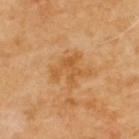{"lighting": "cross-polarized", "image": {"source": "total-body photography crop", "field_of_view_mm": 15}, "patient": {"sex": "male", "age_approx": 70}, "lesion_size": {"long_diameter_mm_approx": 4.0}, "site": "upper back"}